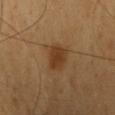Notes:
* notes: imaged on a skin check; not biopsied